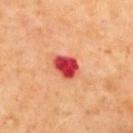| feature | finding |
|---|---|
| notes | imaged on a skin check; not biopsied |
| subject | male, aged around 70 |
| location | the upper back |
| imaging modality | ~15 mm tile from a whole-body skin photo |
| automated metrics | a lesion color around L≈49 a*≈46 b*≈34 in CIELAB and a lesion-to-skin contrast of about 13 (normalized; higher = more distinct); lesion-presence confidence of about 100/100 |